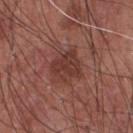Notes:
* follow-up: no biopsy performed (imaged during a skin exam)
* lesion diameter: ~3.5 mm (longest diameter)
* site: the chest
* tile lighting: white-light illumination
* imaging modality: ~15 mm tile from a whole-body skin photo
* automated lesion analysis: a lesion area of about 9 mm², a shape eccentricity near 0.4, and a symmetry-axis asymmetry near 0.3; an average lesion color of about L≈37 a*≈24 b*≈24 (CIELAB) and about 8 CIELAB-L* units darker than the surrounding skin; border irregularity of about 3.5 on a 0–10 scale, a within-lesion color-variation index near 2.5/10, and peripheral color asymmetry of about 1; an automated nevus-likeness rating near 0 out of 100
* patient: male, aged around 65